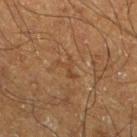Clinical impression: The lesion was tiled from a total-body skin photograph and was not biopsied. Clinical summary: Captured under cross-polarized illumination. Approximately 3 mm at its widest. The lesion is on the left lower leg. A male patient, aged 63 to 67. A lesion tile, about 15 mm wide, cut from a 3D total-body photograph.